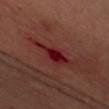The lesion was tiled from a total-body skin photograph and was not biopsied. An algorithmic analysis of the crop reported a lesion area of about 8.5 mm², an outline eccentricity of about 0.9 (0 = round, 1 = elongated), and two-axis asymmetry of about 0.35. It also reported a lesion color around L≈25 a*≈32 b*≈23 in CIELAB, roughly 9 lightness units darker than nearby skin, and a normalized lesion–skin contrast near 9. The analysis additionally found a border-irregularity index near 5/10 and peripheral color asymmetry of about 1. It also reported a detector confidence of about 100 out of 100 that the crop contains a lesion. The patient is a female aged 38–42. Cropped from a whole-body photographic skin survey; the tile spans about 15 mm. Measured at roughly 5.5 mm in maximum diameter. Imaged with cross-polarized lighting. From the left upper arm.